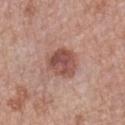Case summary:
• follow-up — catalogued during a skin exam; not biopsied
• acquisition — 15 mm crop, total-body photography
• subject — female, aged 58–62
• automated lesion analysis — an average lesion color of about L≈51 a*≈23 b*≈25 (CIELAB), roughly 12 lightness units darker than nearby skin, and a normalized border contrast of about 8; an automated nevus-likeness rating near 65 out of 100 and a lesion-detection confidence of about 100/100
• lesion size — ~4 mm (longest diameter)
• anatomic site — the left forearm